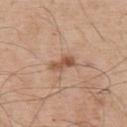Findings:
- workup · catalogued during a skin exam; not biopsied
- anatomic site · the upper back
- automated lesion analysis · a color-variation rating of about 2.5/10 and peripheral color asymmetry of about 0.5
- patient · male, aged approximately 55
- illumination · white-light illumination
- image source · total-body-photography crop, ~15 mm field of view
- size · about 3.5 mm